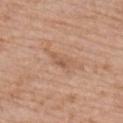Assessment: The lesion was photographed on a routine skin check and not biopsied; there is no pathology result. Context: A male patient, aged 58–62. The lesion is located on the right upper arm. This is a white-light tile. Automated tile analysis of the lesion measured an average lesion color of about L≈57 a*≈20 b*≈32 (CIELAB) and a lesion–skin lightness drop of about 7. And it measured radial color variation of about 1. The analysis additionally found lesion-presence confidence of about 100/100. Longest diameter approximately 3 mm. A lesion tile, about 15 mm wide, cut from a 3D total-body photograph.